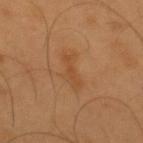A roughly 15 mm field-of-view crop from a total-body skin photograph.
The lesion is located on the upper back.
A male subject, in their mid-50s.